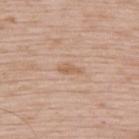| feature | finding |
|---|---|
| biopsy status | total-body-photography surveillance lesion; no biopsy |
| location | the upper back |
| automated metrics | border irregularity of about 4 on a 0–10 scale and a peripheral color-asymmetry measure near 0 |
| patient | male, in their 50s |
| acquisition | ~15 mm tile from a whole-body skin photo |
| lighting | white-light |